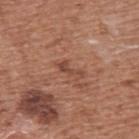Notes:
• workup · no biopsy performed (imaged during a skin exam)
• patient · male, aged 73–77
• tile lighting · white-light illumination
• image source · ~15 mm crop, total-body skin-cancer survey
• anatomic site · the back
• TBP lesion metrics · a classifier nevus-likeness of about 0/100